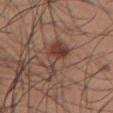notes: imaged on a skin check; not biopsied | anatomic site: the right upper arm | imaging modality: ~15 mm crop, total-body skin-cancer survey | lighting: white-light | lesion size: ≈5.5 mm | patient: male, aged approximately 35.